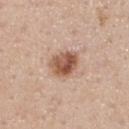Notes:
* notes · catalogued during a skin exam; not biopsied
* lesion diameter · ≈3.5 mm
* image source · ~15 mm crop, total-body skin-cancer survey
* subject · male, in their mid- to late 50s
* location · the chest